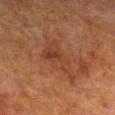biopsy status: imaged on a skin check; not biopsied
patient: male, aged approximately 80
image: total-body-photography crop, ~15 mm field of view
site: the right lower leg
diameter: about 5.5 mm
illumination: cross-polarized